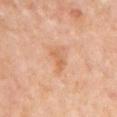The lesion was photographed on a routine skin check and not biopsied; there is no pathology result. Longest diameter approximately 2.5 mm. An algorithmic analysis of the crop reported an automated nevus-likeness rating near 0 out of 100. A region of skin cropped from a whole-body photographic capture, roughly 15 mm wide. The lesion is located on the right upper arm. A female subject, aged around 50.